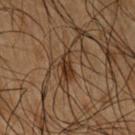notes: catalogued during a skin exam; not biopsied | size: about 2.5 mm | image source: total-body-photography crop, ~15 mm field of view | illumination: cross-polarized illumination | body site: the right upper arm | subject: male, aged approximately 50.